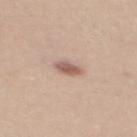notes: catalogued during a skin exam; not biopsied | illumination: white-light | acquisition: total-body-photography crop, ~15 mm field of view | subject: female, aged 23 to 27 | location: the mid back | diameter: ~3 mm (longest diameter) | automated metrics: an area of roughly 3.5 mm², an eccentricity of roughly 0.8, and a shape-asymmetry score of about 0.2 (0 = symmetric); a border-irregularity index near 2/10 and peripheral color asymmetry of about 1.